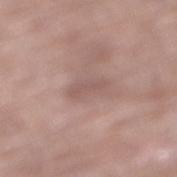| field | value |
|---|---|
| follow-up | imaged on a skin check; not biopsied |
| image source | ~15 mm crop, total-body skin-cancer survey |
| site | the right lower leg |
| patient | female, aged around 80 |
| lighting | white-light illumination |
| image-analysis metrics | a mean CIELAB color near L≈55 a*≈18 b*≈22, about 7 CIELAB-L* units darker than the surrounding skin, and a lesion-to-skin contrast of about 5 (normalized; higher = more distinct) |